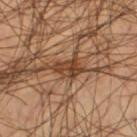notes: catalogued during a skin exam; not biopsied
acquisition: ~15 mm tile from a whole-body skin photo
site: the leg
subject: male, aged around 55